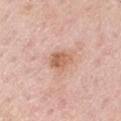Case summary:
– workup: no biopsy performed (imaged during a skin exam)
– size: about 3 mm
– image-analysis metrics: a border-irregularity rating of about 2/10; lesion-presence confidence of about 100/100
– subject: male, aged 58–62
– acquisition: 15 mm crop, total-body photography
– location: the arm
– lighting: white-light illumination